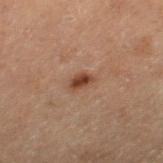The patient is a male approximately 70 years of age. From the left lower leg. A lesion tile, about 15 mm wide, cut from a 3D total-body photograph. Measured at roughly 2.5 mm in maximum diameter.